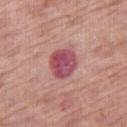<case>
<biopsy_status>not biopsied; imaged during a skin examination</biopsy_status>
<lesion_size>
  <long_diameter_mm_approx>4.0</long_diameter_mm_approx>
</lesion_size>
<lighting>white-light</lighting>
<automated_metrics>
  <area_mm2_approx>10.0</area_mm2_approx>
  <eccentricity>0.45</eccentricity>
  <shape_asymmetry>0.15</shape_asymmetry>
  <lesion_detection_confidence_0_100>100</lesion_detection_confidence_0_100>
</automated_metrics>
<patient>
  <sex>male</sex>
  <age_approx>70</age_approx>
</patient>
<image>
  <source>total-body photography crop</source>
  <field_of_view_mm>15</field_of_view_mm>
</image>
<site>right thigh</site>
</case>